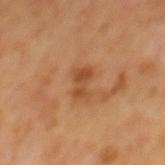Part of a total-body skin-imaging series; this lesion was reviewed on a skin check and was not flagged for biopsy. A 15 mm crop from a total-body photograph taken for skin-cancer surveillance. A male patient roughly 65 years of age. The lesion is on the mid back.